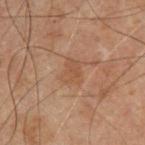Assessment: The lesion was photographed on a routine skin check and not biopsied; there is no pathology result. Acquisition and patient details: This is a cross-polarized tile. Located on the chest. Approximately 2.5 mm at its widest. A 15 mm crop from a total-body photograph taken for skin-cancer surveillance. The subject is a male aged 68–72.